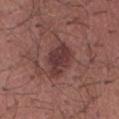• workup: no biopsy performed (imaged during a skin exam)
• automated metrics: an outline eccentricity of about 0.75 (0 = round, 1 = elongated) and a shape-asymmetry score of about 0.2 (0 = symmetric); an average lesion color of about L≈37 a*≈21 b*≈19 (CIELAB), a lesion–skin lightness drop of about 9, and a normalized lesion–skin contrast near 8.5; a border-irregularity index near 2.5/10, internal color variation of about 3 on a 0–10 scale, and a peripheral color-asymmetry measure near 1
• lighting: white-light
• diameter: ~5 mm (longest diameter)
• acquisition: 15 mm crop, total-body photography
• patient: male, about 55 years old
• location: the abdomen